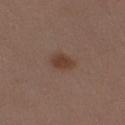Q: Is there a histopathology result?
A: imaged on a skin check; not biopsied
Q: What kind of image is this?
A: ~15 mm crop, total-body skin-cancer survey
Q: Patient demographics?
A: female, aged approximately 40
Q: Where on the body is the lesion?
A: the left thigh